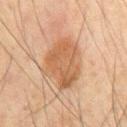Q: Is there a histopathology result?
A: no biopsy performed (imaged during a skin exam)
Q: What did automated image analysis measure?
A: an average lesion color of about L≈47 a*≈17 b*≈30 (CIELAB), about 9 CIELAB-L* units darker than the surrounding skin, and a normalized lesion–skin contrast near 7.5; border irregularity of about 3 on a 0–10 scale, internal color variation of about 3.5 on a 0–10 scale, and peripheral color asymmetry of about 1
Q: How large is the lesion?
A: ≈7 mm
Q: What is the imaging modality?
A: ~15 mm crop, total-body skin-cancer survey
Q: Illumination type?
A: cross-polarized
Q: Lesion location?
A: the chest
Q: Who is the patient?
A: male, aged 63–67